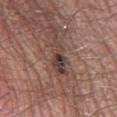Captured during whole-body skin photography for melanoma surveillance; the lesion was not biopsied.
A 15 mm crop from a total-body photograph taken for skin-cancer surveillance.
Automated image analysis of the tile measured a border-irregularity rating of about 5/10, a within-lesion color-variation index near 7.5/10, and peripheral color asymmetry of about 2.5.
Approximately 5.5 mm at its widest.
Captured under white-light illumination.
The patient is a male about 55 years old.
On the left thigh.